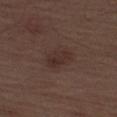No biopsy was performed on this lesion — it was imaged during a full skin examination and was not determined to be concerning. Imaged with white-light lighting. A male subject, aged 68 to 72. Longest diameter approximately 3.5 mm. Automated image analysis of the tile measured a lesion area of about 6 mm², a shape eccentricity near 0.8, and two-axis asymmetry of about 0.15. It also reported a within-lesion color-variation index near 2.5/10 and peripheral color asymmetry of about 1. Located on the left thigh. A 15 mm close-up tile from a total-body photography series done for melanoma screening.